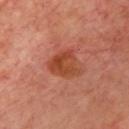Impression: Captured during whole-body skin photography for melanoma surveillance; the lesion was not biopsied. Image and clinical context: The lesion is on the chest. A lesion tile, about 15 mm wide, cut from a 3D total-body photograph. The total-body-photography lesion software estimated border irregularity of about 4 on a 0–10 scale, a color-variation rating of about 4.5/10, and radial color variation of about 1.5. Imaged with cross-polarized lighting. Measured at roughly 4 mm in maximum diameter. A male subject, about 65 years old.